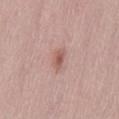{"biopsy_status": "not biopsied; imaged during a skin examination", "image": {"source": "total-body photography crop", "field_of_view_mm": 15}, "patient": {"sex": "male", "age_approx": 60}, "automated_metrics": {"area_mm2_approx": 3.5, "eccentricity": 0.8, "shape_asymmetry": 0.3, "cielab_L": 56, "cielab_a": 22, "cielab_b": 25, "vs_skin_darker_L": 10.0, "vs_skin_contrast_norm": 7.0, "peripheral_color_asymmetry": 0.5}, "site": "lower back"}